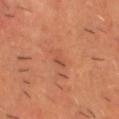<case>
<biopsy_status>not biopsied; imaged during a skin examination</biopsy_status>
<automated_metrics>
  <area_mm2_approx>2.0</area_mm2_approx>
  <cielab_L>46</cielab_L>
  <cielab_a>25</cielab_a>
  <cielab_b>32</cielab_b>
  <vs_skin_darker_L>6.0</vs_skin_darker_L>
  <vs_skin_contrast_norm>5.0</vs_skin_contrast_norm>
  <nevus_likeness_0_100>0</nevus_likeness_0_100>
  <lesion_detection_confidence_0_100>90</lesion_detection_confidence_0_100>
</automated_metrics>
<image>
  <source>total-body photography crop</source>
  <field_of_view_mm>15</field_of_view_mm>
</image>
<site>head or neck</site>
<patient>
  <sex>male</sex>
  <age_approx>65</age_approx>
</patient>
<lesion_size>
  <long_diameter_mm_approx>2.5</long_diameter_mm_approx>
</lesion_size>
</case>